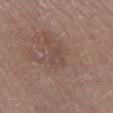The lesion was photographed on a routine skin check and not biopsied; there is no pathology result. Located on the left lower leg. The recorded lesion diameter is about 2.5 mm. A 15 mm close-up tile from a total-body photography series done for melanoma screening. Automated image analysis of the tile measured an average lesion color of about L≈46 a*≈17 b*≈22 (CIELAB), a lesion–skin lightness drop of about 4, and a normalized border contrast of about 3.5. It also reported a border-irregularity index near 2.5/10, a color-variation rating of about 2/10, and radial color variation of about 0.5. The tile uses white-light illumination. A male patient, aged 63–67.